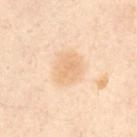Findings:
* anatomic site: the abdomen
* patient: female, aged 53 to 57
* image-analysis metrics: a lesion area of about 10 mm², a shape eccentricity near 0.35, and a shape-asymmetry score of about 0.15 (0 = symmetric); a lesion–skin lightness drop of about 6 and a normalized lesion–skin contrast near 5; a border-irregularity rating of about 1.5/10; a lesion-detection confidence of about 100/100
* imaging modality: total-body-photography crop, ~15 mm field of view